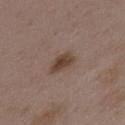lighting: white-light illumination; subject: female, in their mid-30s; body site: the upper back; lesion size: ≈3.5 mm; TBP lesion metrics: a border-irregularity rating of about 2/10; image: 15 mm crop, total-body photography.